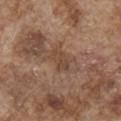The lesion was tiled from a total-body skin photograph and was not biopsied.